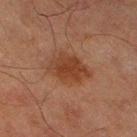Imaged during a routine full-body skin examination; the lesion was not biopsied and no histopathology is available.
Captured under cross-polarized illumination.
An algorithmic analysis of the crop reported a mean CIELAB color near L≈32 a*≈19 b*≈27 and roughly 8 lightness units darker than nearby skin. The software also gave a border-irregularity index near 3/10 and a color-variation rating of about 3/10. It also reported a nevus-likeness score of about 60/100 and lesion-presence confidence of about 100/100.
The recorded lesion diameter is about 4.5 mm.
On the left thigh.
Cropped from a whole-body photographic skin survey; the tile spans about 15 mm.
A male subject, about 65 years old.